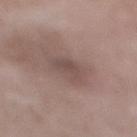patient: male, roughly 50 years of age
tile lighting: white-light
site: the right lower leg
image: total-body-photography crop, ~15 mm field of view
size: ≈4 mm